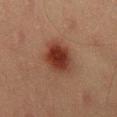Findings:
- follow-up · imaged on a skin check; not biopsied
- tile lighting · cross-polarized
- imaging modality · ~15 mm tile from a whole-body skin photo
- automated lesion analysis · a footprint of about 12 mm², an outline eccentricity of about 0.6 (0 = round, 1 = elongated), and a shape-asymmetry score of about 0.15 (0 = symmetric); a lesion color around L≈29 a*≈21 b*≈25 in CIELAB, a lesion–skin lightness drop of about 11, and a lesion-to-skin contrast of about 11 (normalized; higher = more distinct)
- anatomic site · the right thigh
- patient · male, roughly 40 years of age